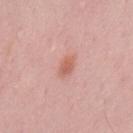image source: total-body-photography crop, ~15 mm field of view | lesion diameter: about 2.5 mm | subject: male, about 50 years old | automated lesion analysis: a footprint of about 4 mm² and an outline eccentricity of about 0.8 (0 = round, 1 = elongated); a lesion-to-skin contrast of about 6.5 (normalized; higher = more distinct); a border-irregularity rating of about 2.5/10, a color-variation rating of about 1.5/10, and a peripheral color-asymmetry measure near 0.5; a lesion-detection confidence of about 100/100 | tile lighting: white-light | body site: the mid back.